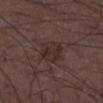{"biopsy_status": "not biopsied; imaged during a skin examination", "site": "right lower leg", "lighting": "white-light", "lesion_size": {"long_diameter_mm_approx": 3.5}, "image": {"source": "total-body photography crop", "field_of_view_mm": 15}, "patient": {"sex": "male", "age_approx": 50}}